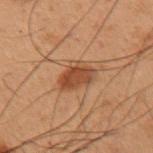workup: catalogued during a skin exam; not biopsied | subject: male, about 55 years old | lighting: cross-polarized illumination | size: ≈3.5 mm | acquisition: total-body-photography crop, ~15 mm field of view | TBP lesion metrics: a lesion area of about 8.5 mm², an outline eccentricity of about 0.65 (0 = round, 1 = elongated), and a symmetry-axis asymmetry near 0.25; roughly 9 lightness units darker than nearby skin and a normalized border contrast of about 8; border irregularity of about 2.5 on a 0–10 scale, internal color variation of about 3 on a 0–10 scale, and a peripheral color-asymmetry measure near 1 | site: the left upper arm.